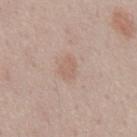Clinical impression: Captured during whole-body skin photography for melanoma surveillance; the lesion was not biopsied. Image and clinical context: A roughly 15 mm field-of-view crop from a total-body skin photograph. The lesion is on the abdomen. A male subject, aged around 60. The tile uses white-light illumination. The recorded lesion diameter is about 2.5 mm.